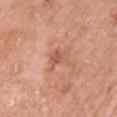<lesion>
  <biopsy_status>not biopsied; imaged during a skin examination</biopsy_status>
  <site>chest</site>
  <patient>
    <sex>female</sex>
    <age_approx>60</age_approx>
  </patient>
  <image>
    <source>total-body photography crop</source>
    <field_of_view_mm>15</field_of_view_mm>
  </image>
</lesion>